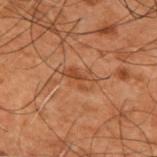patient: male, approximately 50 years of age
location: the upper back
automated metrics: a mean CIELAB color near L≈35 a*≈20 b*≈29, roughly 6 lightness units darker than nearby skin, and a normalized lesion–skin contrast near 6
imaging modality: ~15 mm crop, total-body skin-cancer survey